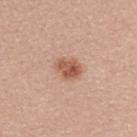Clinical summary:
The subject is a female aged around 25. A region of skin cropped from a whole-body photographic capture, roughly 15 mm wide. About 3 mm across. From the upper back.